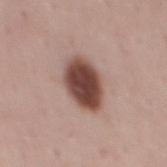Imaged during a routine full-body skin examination; the lesion was not biopsied and no histopathology is available. Measured at roughly 5.5 mm in maximum diameter. The lesion is on the mid back. Imaged with white-light lighting. A roughly 15 mm field-of-view crop from a total-body skin photograph. A male subject approximately 45 years of age.